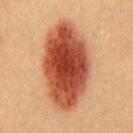Imaged during a routine full-body skin examination; the lesion was not biopsied and no histopathology is available.
From the chest.
A lesion tile, about 15 mm wide, cut from a 3D total-body photograph.
Automated tile analysis of the lesion measured an average lesion color of about L≈40 a*≈26 b*≈30 (CIELAB) and a lesion–skin lightness drop of about 17. The software also gave border irregularity of about 2 on a 0–10 scale and a color-variation rating of about 6.5/10.
Approximately 10.5 mm at its widest.
A male subject aged 38–42.
This is a cross-polarized tile.